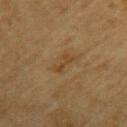{"biopsy_status": "not biopsied; imaged during a skin examination", "site": "left upper arm", "lesion_size": {"long_diameter_mm_approx": 3.0}, "lighting": "cross-polarized", "image": {"source": "total-body photography crop", "field_of_view_mm": 15}, "automated_metrics": {"area_mm2_approx": 3.5, "eccentricity": 0.9, "shape_asymmetry": 0.3, "cielab_L": 37, "cielab_a": 14, "cielab_b": 31, "vs_skin_darker_L": 5.0, "vs_skin_contrast_norm": 5.5, "border_irregularity_0_10": 4.5, "color_variation_0_10": 0.0, "peripheral_color_asymmetry": 0.0, "nevus_likeness_0_100": 0, "lesion_detection_confidence_0_100": 95}, "patient": {"sex": "male", "age_approx": "85+"}}